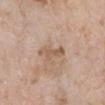This lesion was catalogued during total-body skin photography and was not selected for biopsy. From the right lower leg. Captured under white-light illumination. A female patient, aged approximately 70. A 15 mm close-up tile from a total-body photography series done for melanoma screening. About 4 mm across.